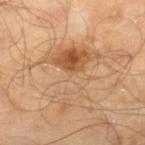<tbp_lesion>
<lesion_size>
  <long_diameter_mm_approx>8.0</long_diameter_mm_approx>
</lesion_size>
<patient>
  <sex>male</sex>
  <age_approx>65</age_approx>
</patient>
<image>
  <source>total-body photography crop</source>
  <field_of_view_mm>15</field_of_view_mm>
</image>
<site>right lower leg</site>
<lighting>cross-polarized</lighting>
</tbp_lesion>